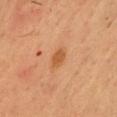Part of a total-body skin-imaging series; this lesion was reviewed on a skin check and was not flagged for biopsy.
The patient is a male aged around 35.
The lesion is located on the front of the torso.
The total-body-photography lesion software estimated a lesion color around L≈51 a*≈23 b*≈39 in CIELAB, roughly 8 lightness units darker than nearby skin, and a normalized border contrast of about 7.
Imaged with cross-polarized lighting.
Cropped from a whole-body photographic skin survey; the tile spans about 15 mm.
Measured at roughly 3 mm in maximum diameter.